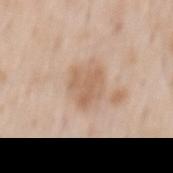notes: catalogued during a skin exam; not biopsied
subject: male, aged around 60
automated lesion analysis: a lesion area of about 8.5 mm² and an outline eccentricity of about 0.55 (0 = round, 1 = elongated); an average lesion color of about L≈61 a*≈18 b*≈31 (CIELAB), roughly 9 lightness units darker than nearby skin, and a lesion-to-skin contrast of about 6.5 (normalized; higher = more distinct); a nevus-likeness score of about 0/100 and lesion-presence confidence of about 100/100
tile lighting: white-light
acquisition: ~15 mm crop, total-body skin-cancer survey
location: the mid back
lesion diameter: about 3.5 mm